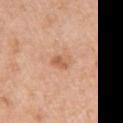Notes:
• workup · imaged on a skin check; not biopsied
• subject · female, approximately 40 years of age
• size · ≈3 mm
• automated metrics · an average lesion color of about L≈61 a*≈23 b*≈35 (CIELAB) and a lesion–skin lightness drop of about 9; a classifier nevus-likeness of about 5/100 and a detector confidence of about 100 out of 100 that the crop contains a lesion
• anatomic site · the left upper arm
• lighting · white-light
• acquisition · 15 mm crop, total-body photography